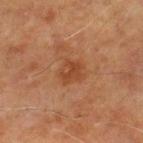{"biopsy_status": "not biopsied; imaged during a skin examination", "image": {"source": "total-body photography crop", "field_of_view_mm": 15}, "site": "leg", "patient": {"sex": "male", "age_approx": 70}}